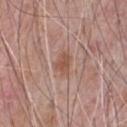Image and clinical context: On the chest. Imaged with white-light lighting. The subject is a male aged around 70. The lesion's longest dimension is about 2.5 mm. A 15 mm crop from a total-body photograph taken for skin-cancer surveillance.